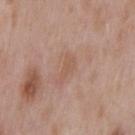This lesion was catalogued during total-body skin photography and was not selected for biopsy. A male subject, aged approximately 55. Cropped from a whole-body photographic skin survey; the tile spans about 15 mm. Longest diameter approximately 3.5 mm. Captured under white-light illumination. Located on the back.